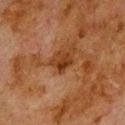Q: What did automated image analysis measure?
A: a mean CIELAB color near L≈29 a*≈20 b*≈30, about 9 CIELAB-L* units darker than the surrounding skin, and a normalized lesion–skin contrast near 9
Q: What is the imaging modality?
A: total-body-photography crop, ~15 mm field of view
Q: Who is the patient?
A: male, in their 80s
Q: What is the lesion's diameter?
A: ≈2.5 mm
Q: What is the anatomic site?
A: the upper back
Q: How was the tile lit?
A: cross-polarized